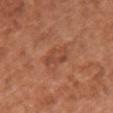Imaged during a routine full-body skin examination; the lesion was not biopsied and no histopathology is available. The recorded lesion diameter is about 3.5 mm. An algorithmic analysis of the crop reported a lesion area of about 6 mm², an outline eccentricity of about 0.8 (0 = round, 1 = elongated), and two-axis asymmetry of about 0.25. It also reported a mean CIELAB color near L≈47 a*≈26 b*≈33, a lesion–skin lightness drop of about 7, and a lesion-to-skin contrast of about 5.5 (normalized; higher = more distinct). The tile uses white-light illumination. The lesion is located on the chest. Cropped from a whole-body photographic skin survey; the tile spans about 15 mm. The patient is a female roughly 65 years of age.